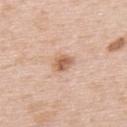lighting=white-light; TBP lesion metrics=a classifier nevus-likeness of about 80/100 and a detector confidence of about 100 out of 100 that the crop contains a lesion; location=the upper back; diameter=~3 mm (longest diameter); subject=male, roughly 50 years of age; imaging modality=15 mm crop, total-body photography.